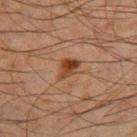Clinical summary:
On the right thigh. A 15 mm close-up extracted from a 3D total-body photography capture. The subject is a male about 65 years old.